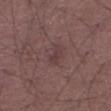Clinical impression:
Recorded during total-body skin imaging; not selected for excision or biopsy.
Context:
An algorithmic analysis of the crop reported a color-variation rating of about 0/10. A male patient, approximately 60 years of age. Cropped from a total-body skin-imaging series; the visible field is about 15 mm. Captured under white-light illumination. Approximately 3 mm at its widest. The lesion is on the right thigh.